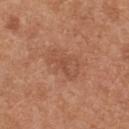  biopsy_status: not biopsied; imaged during a skin examination
  site: upper back
  lighting: white-light
  lesion_size:
    long_diameter_mm_approx: 4.0
  patient:
    sex: female
    age_approx: 40
  image:
    source: total-body photography crop
    field_of_view_mm: 15